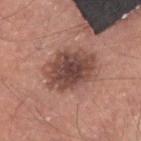image source = ~15 mm crop, total-body skin-cancer survey | automated metrics = an eccentricity of roughly 0.7 and two-axis asymmetry of about 0.15; an average lesion color of about L≈46 a*≈21 b*≈24 (CIELAB); a border-irregularity index near 2.5/10 and radial color variation of about 1.5 | body site = the head or neck | subject = male, roughly 60 years of age | lighting = white-light | pathology = a dysplastic (Clark) nevus.